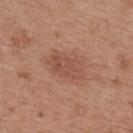{
  "biopsy_status": "not biopsied; imaged during a skin examination",
  "lighting": "white-light",
  "image": {
    "source": "total-body photography crop",
    "field_of_view_mm": 15
  },
  "lesion_size": {
    "long_diameter_mm_approx": 5.0
  },
  "site": "upper back",
  "patient": {
    "sex": "female",
    "age_approx": 40
  }
}